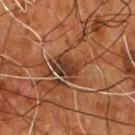body site — the chest
subject — male, aged around 55
image — 15 mm crop, total-body photography